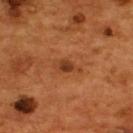This lesion was catalogued during total-body skin photography and was not selected for biopsy.
About 3.5 mm across.
On the upper back.
Captured under cross-polarized illumination.
The subject is a male aged approximately 55.
A lesion tile, about 15 mm wide, cut from a 3D total-body photograph.